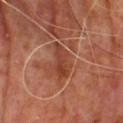Part of a total-body skin-imaging series; this lesion was reviewed on a skin check and was not flagged for biopsy.
The lesion is on the upper back.
Measured at roughly 4.5 mm in maximum diameter.
Automated tile analysis of the lesion measured an outline eccentricity of about 0.85 (0 = round, 1 = elongated) and a shape-asymmetry score of about 0.4 (0 = symmetric). And it measured an average lesion color of about L≈40 a*≈26 b*≈30 (CIELAB), a lesion–skin lightness drop of about 8, and a lesion-to-skin contrast of about 7 (normalized; higher = more distinct). The software also gave a lesion-detection confidence of about 100/100.
A male patient about 60 years old.
A lesion tile, about 15 mm wide, cut from a 3D total-body photograph.